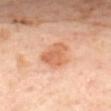follow-up = imaged on a skin check; not biopsied